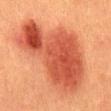Captured during whole-body skin photography for melanoma surveillance; the lesion was not biopsied. A 15 mm close-up extracted from a 3D total-body photography capture. Automated tile analysis of the lesion measured a border-irregularity index near 5/10, a within-lesion color-variation index near 7/10, and peripheral color asymmetry of about 2.5. About 10.5 mm across. This is a cross-polarized tile. Located on the back. A male subject approximately 40 years of age.